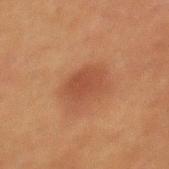Assessment: The lesion was photographed on a routine skin check and not biopsied; there is no pathology result. Image and clinical context: The patient is a male about 50 years old. From the mid back. Cropped from a whole-body photographic skin survey; the tile spans about 15 mm.